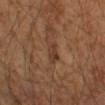Imaged during a routine full-body skin examination; the lesion was not biopsied and no histopathology is available. The subject is a male aged approximately 65. Located on the arm. A region of skin cropped from a whole-body photographic capture, roughly 15 mm wide.